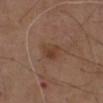Assessment:
Imaged during a routine full-body skin examination; the lesion was not biopsied and no histopathology is available.
Background:
This image is a 15 mm lesion crop taken from a total-body photograph. A male subject approximately 75 years of age. The lesion is on the chest. An algorithmic analysis of the crop reported a footprint of about 6 mm². It also reported a mean CIELAB color near L≈40 a*≈19 b*≈28 and a normalized lesion–skin contrast near 6.5. And it measured radial color variation of about 1. Measured at roughly 3 mm in maximum diameter.